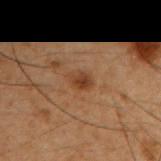Clinical impression:
Imaged during a routine full-body skin examination; the lesion was not biopsied and no histopathology is available.
Clinical summary:
The lesion is on the right upper arm. A 15 mm crop from a total-body photograph taken for skin-cancer surveillance. The tile uses cross-polarized illumination. Approximately 3 mm at its widest. A male patient in their 50s.